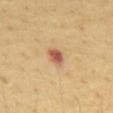Captured during whole-body skin photography for melanoma surveillance; the lesion was not biopsied. On the mid back. This is a cross-polarized tile. The recorded lesion diameter is about 2.5 mm. The subject is a male aged approximately 65. Cropped from a whole-body photographic skin survey; the tile spans about 15 mm.